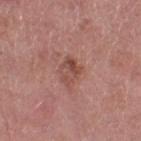Notes:
• workup · imaged on a skin check; not biopsied
• subject · female, roughly 70 years of age
• tile lighting · white-light
• automated lesion analysis · a lesion color around L≈48 a*≈23 b*≈25 in CIELAB, roughly 9 lightness units darker than nearby skin, and a normalized border contrast of about 6.5; a nevus-likeness score of about 5/100 and a lesion-detection confidence of about 100/100
• acquisition · ~15 mm crop, total-body skin-cancer survey
• anatomic site · the left thigh
• diameter · about 3 mm